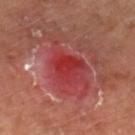A lesion tile, about 15 mm wide, cut from a 3D total-body photograph.
A female patient, aged approximately 60.
On the left leg.
The biopsy diagnosis was an actinic keratosis (borderline).